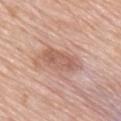notes: total-body-photography surveillance lesion; no biopsy
size: ~6 mm (longest diameter)
image source: total-body-photography crop, ~15 mm field of view
tile lighting: white-light illumination
subject: male, roughly 80 years of age
site: the back